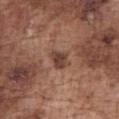  biopsy_status: not biopsied; imaged during a skin examination
  image:
    source: total-body photography crop
    field_of_view_mm: 15
  lighting: white-light
  site: chest
  patient:
    sex: male
    age_approx: 75